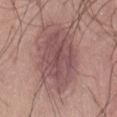{"automated_metrics": {"area_mm2_approx": 26.0, "eccentricity": 0.75, "shape_asymmetry": 0.2}, "image": {"source": "total-body photography crop", "field_of_view_mm": 15}, "site": "abdomen", "patient": {"sex": "male", "age_approx": 60}}